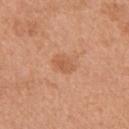No biopsy was performed on this lesion — it was imaged during a full skin examination and was not determined to be concerning. The lesion is located on the left upper arm. A 15 mm crop from a total-body photograph taken for skin-cancer surveillance. The patient is a female aged 58–62. Automated tile analysis of the lesion measured a footprint of about 3.5 mm² and a symmetry-axis asymmetry near 0.25.